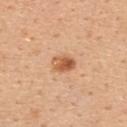Recorded during total-body skin imaging; not selected for excision or biopsy. Measured at roughly 3 mm in maximum diameter. Automated image analysis of the tile measured a lesion area of about 5.5 mm² and a shape-asymmetry score of about 0.15 (0 = symmetric). The software also gave an average lesion color of about L≈59 a*≈25 b*≈38 (CIELAB) and a lesion-to-skin contrast of about 8.5 (normalized; higher = more distinct). The analysis additionally found a nevus-likeness score of about 95/100 and a lesion-detection confidence of about 100/100. This image is a 15 mm lesion crop taken from a total-body photograph. The lesion is on the upper back. A female subject, approximately 45 years of age. The tile uses white-light illumination.